A male subject roughly 55 years of age · a region of skin cropped from a whole-body photographic capture, roughly 15 mm wide · Automated image analysis of the tile measured a footprint of about 38 mm², a shape eccentricity near 0.8, and a symmetry-axis asymmetry near 0.4. The analysis additionally found an average lesion color of about L≈21 a*≈12 b*≈15 (CIELAB), roughly 6 lightness units darker than nearby skin, and a lesion-to-skin contrast of about 8 (normalized; higher = more distinct). The analysis additionally found internal color variation of about 3 on a 0–10 scale · the tile uses white-light illumination · the lesion is located on the left thigh · about 9.5 mm across: 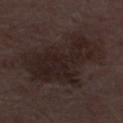On biopsy, histopathology showed a seborrheic keratosis, classified as a benign skin lesion.